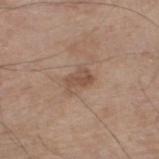Assessment: The lesion was photographed on a routine skin check and not biopsied; there is no pathology result. Acquisition and patient details: The lesion's longest dimension is about 3 mm. On the left thigh. A roughly 15 mm field-of-view crop from a total-body skin photograph. A male subject, aged 78 to 82.